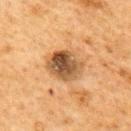The lesion was photographed on a routine skin check and not biopsied; there is no pathology result. A female patient, roughly 60 years of age. A close-up tile cropped from a whole-body skin photograph, about 15 mm across. The lesion's longest dimension is about 4 mm. From the upper back. This is a cross-polarized tile.